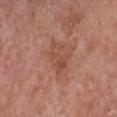The lesion was photographed on a routine skin check and not biopsied; there is no pathology result. The recorded lesion diameter is about 3.5 mm. A female subject, approximately 60 years of age. The lesion-visualizer software estimated a mean CIELAB color near L≈49 a*≈24 b*≈29, a lesion–skin lightness drop of about 7, and a lesion-to-skin contrast of about 5.5 (normalized; higher = more distinct). The analysis additionally found a border-irregularity rating of about 4.5/10, internal color variation of about 3.5 on a 0–10 scale, and radial color variation of about 1.5. On the chest. Cropped from a whole-body photographic skin survey; the tile spans about 15 mm.